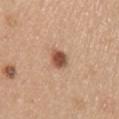Imaged during a routine full-body skin examination; the lesion was not biopsied and no histopathology is available.
A 15 mm close-up tile from a total-body photography series done for melanoma screening.
This is a white-light tile.
The lesion is on the right upper arm.
A female patient, aged approximately 65.
Longest diameter approximately 2.5 mm.
The lesion-visualizer software estimated an average lesion color of about L≈52 a*≈22 b*≈31 (CIELAB), a lesion–skin lightness drop of about 16, and a lesion-to-skin contrast of about 10.5 (normalized; higher = more distinct). The software also gave a border-irregularity rating of about 1.5/10 and peripheral color asymmetry of about 1. The analysis additionally found a nevus-likeness score of about 100/100 and a detector confidence of about 100 out of 100 that the crop contains a lesion.